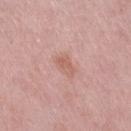follow-up: no biopsy performed (imaged during a skin exam) | diameter: ~3 mm (longest diameter) | image-analysis metrics: a footprint of about 3.5 mm², an outline eccentricity of about 0.85 (0 = round, 1 = elongated), and two-axis asymmetry of about 0.3; a border-irregularity index near 3/10, internal color variation of about 1.5 on a 0–10 scale, and a peripheral color-asymmetry measure near 0.5; a nevus-likeness score of about 5/100 | body site: the right thigh | tile lighting: white-light | subject: female, approximately 40 years of age | image: ~15 mm crop, total-body skin-cancer survey.